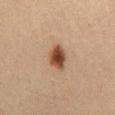Findings:
* biopsy status: total-body-photography surveillance lesion; no biopsy
* subject: male, in their mid-30s
* location: the abdomen
* image: total-body-photography crop, ~15 mm field of view
* illumination: cross-polarized illumination